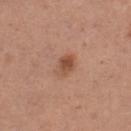  biopsy_status: not biopsied; imaged during a skin examination
  lesion_size:
    long_diameter_mm_approx: 3.0
  site: left thigh
  lighting: white-light
  automated_metrics:
    area_mm2_approx: 4.5
    eccentricity: 0.8
    shape_asymmetry: 0.2
    cielab_L: 51
    cielab_a: 23
    cielab_b: 31
    vs_skin_darker_L: 10.0
    color_variation_0_10: 4.0
    peripheral_color_asymmetry: 1.5
    nevus_likeness_0_100: 85
    lesion_detection_confidence_0_100: 100
  image:
    source: total-body photography crop
    field_of_view_mm: 15
  patient:
    sex: female
    age_approx: 30